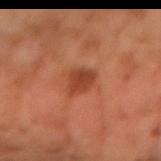Q: Was a biopsy performed?
A: total-body-photography surveillance lesion; no biopsy
Q: Illumination type?
A: cross-polarized illumination
Q: Patient demographics?
A: male, aged 63 to 67
Q: Automated lesion metrics?
A: two-axis asymmetry of about 0.25; a border-irregularity index near 2.5/10 and radial color variation of about 1; an automated nevus-likeness rating near 75 out of 100
Q: How was this image acquired?
A: ~15 mm tile from a whole-body skin photo